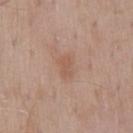The tile uses white-light illumination. Cropped from a whole-body photographic skin survey; the tile spans about 15 mm. Located on the mid back. The lesion's longest dimension is about 3 mm. A male subject in their mid-50s.